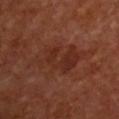The lesion was tiled from a total-body skin photograph and was not biopsied.
A female subject, aged 63–67.
This image is a 15 mm lesion crop taken from a total-body photograph.
From the front of the torso.